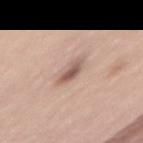Captured during whole-body skin photography for melanoma surveillance; the lesion was not biopsied. An algorithmic analysis of the crop reported a mean CIELAB color near L≈57 a*≈19 b*≈25, about 13 CIELAB-L* units darker than the surrounding skin, and a normalized lesion–skin contrast near 8. The lesion is on the lower back. A male patient, in their mid- to late 60s. A 15 mm close-up tile from a total-body photography series done for melanoma screening. Approximately 3 mm at its widest.